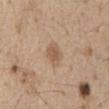A male patient, about 70 years old. Measured at roughly 3 mm in maximum diameter. The lesion is located on the front of the torso. The lesion-visualizer software estimated a lesion color around L≈57 a*≈17 b*≈31 in CIELAB, roughly 9 lightness units darker than nearby skin, and a normalized lesion–skin contrast near 6.5. It also reported radial color variation of about 1. It also reported a nevus-likeness score of about 75/100 and a detector confidence of about 100 out of 100 that the crop contains a lesion. A close-up tile cropped from a whole-body skin photograph, about 15 mm across.